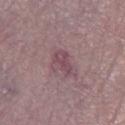{
  "biopsy_status": "not biopsied; imaged during a skin examination",
  "site": "right lower leg",
  "lighting": "white-light",
  "patient": {
    "sex": "female",
    "age_approx": 70
  },
  "image": {
    "source": "total-body photography crop",
    "field_of_view_mm": 15
  },
  "lesion_size": {
    "long_diameter_mm_approx": 3.5
  }
}